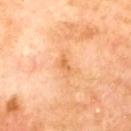The lesion is located on the upper back.
The subject is a male in their 70s.
This image is a 15 mm lesion crop taken from a total-body photograph.